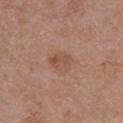<lesion>
  <biopsy_status>not biopsied; imaged during a skin examination</biopsy_status>
  <patient>
    <sex>female</sex>
    <age_approx>50</age_approx>
  </patient>
  <site>chest</site>
  <image>
    <source>total-body photography crop</source>
    <field_of_view_mm>15</field_of_view_mm>
  </image>
</lesion>